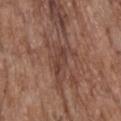| key | value |
|---|---|
| notes | no biopsy performed (imaged during a skin exam) |
| image source | ~15 mm tile from a whole-body skin photo |
| lighting | white-light illumination |
| subject | male, aged 68 to 72 |
| location | the upper back |
| size | ~3 mm (longest diameter) |